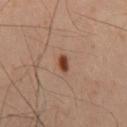Assessment: Part of a total-body skin-imaging series; this lesion was reviewed on a skin check and was not flagged for biopsy. Context: The lesion is on the mid back. A male patient aged around 45. About 2.5 mm across. A region of skin cropped from a whole-body photographic capture, roughly 15 mm wide. Captured under cross-polarized illumination.